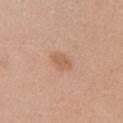Q: Was a biopsy performed?
A: imaged on a skin check; not biopsied